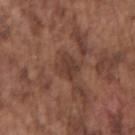• follow-up · catalogued during a skin exam; not biopsied
• acquisition · 15 mm crop, total-body photography
• tile lighting · white-light
• patient · male, aged approximately 75
• location · the arm